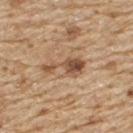The lesion was photographed on a routine skin check and not biopsied; there is no pathology result.
A male patient aged around 70.
This image is a 15 mm lesion crop taken from a total-body photograph.
The lesion is on the upper back.